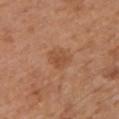biopsy_status: not biopsied; imaged during a skin examination
automated_metrics:
  area_mm2_approx: 5.0
  eccentricity: 0.55
  shape_asymmetry: 0.25
  border_irregularity_0_10: 2.0
  color_variation_0_10: 1.5
  peripheral_color_asymmetry: 0.5
patient:
  sex: male
  age_approx: 65
lighting: white-light
image:
  source: total-body photography crop
  field_of_view_mm: 15
lesion_size:
  long_diameter_mm_approx: 2.5
site: left upper arm